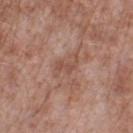{
  "biopsy_status": "not biopsied; imaged during a skin examination"
}